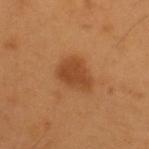Imaged during a routine full-body skin examination; the lesion was not biopsied and no histopathology is available.
The subject is a male about 55 years old.
On the back.
Measured at roughly 4 mm in maximum diameter.
This is a cross-polarized tile.
Cropped from a total-body skin-imaging series; the visible field is about 15 mm.